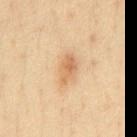  biopsy_status: not biopsied; imaged during a skin examination
  patient:
    sex: male
    age_approx: 35
  image:
    source: total-body photography crop
    field_of_view_mm: 15
  site: abdomen